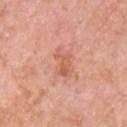Notes:
- biopsy status — total-body-photography surveillance lesion; no biopsy
- lesion diameter — about 3 mm
- illumination — white-light
- location — the chest
- image source — 15 mm crop, total-body photography
- subject — male, aged approximately 80